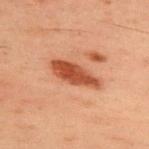biopsy status — catalogued during a skin exam; not biopsied
site — the upper back
acquisition — 15 mm crop, total-body photography
lesion size — about 5.5 mm
patient — male, aged 58 to 62